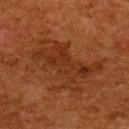Impression:
Recorded during total-body skin imaging; not selected for excision or biopsy.
Clinical summary:
A female patient, aged around 50. A region of skin cropped from a whole-body photographic capture, roughly 15 mm wide. From the upper back.